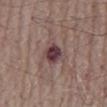The lesion was tiled from a total-body skin photograph and was not biopsied.
The lesion is located on the abdomen.
A 15 mm close-up tile from a total-body photography series done for melanoma screening.
Measured at roughly 4 mm in maximum diameter.
An algorithmic analysis of the crop reported a lesion area of about 8.5 mm² and an eccentricity of roughly 0.65. And it measured a lesion color around L≈41 a*≈18 b*≈16 in CIELAB, about 12 CIELAB-L* units darker than the surrounding skin, and a normalized border contrast of about 10. And it measured a border-irregularity rating of about 4/10, a within-lesion color-variation index near 6/10, and peripheral color asymmetry of about 1.5. The analysis additionally found an automated nevus-likeness rating near 25 out of 100 and a lesion-detection confidence of about 100/100.
Imaged with white-light lighting.
A male patient aged around 80.